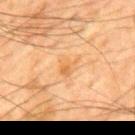No biopsy was performed on this lesion — it was imaged during a full skin examination and was not determined to be concerning.
A roughly 15 mm field-of-view crop from a total-body skin photograph.
The subject is a male aged 63 to 67.
About 3 mm across.
The lesion is located on the mid back.
The total-body-photography lesion software estimated an average lesion color of about L≈54 a*≈19 b*≈38 (CIELAB) and roughly 6 lightness units darker than nearby skin. The analysis additionally found border irregularity of about 3.5 on a 0–10 scale, a within-lesion color-variation index near 3/10, and peripheral color asymmetry of about 1. The software also gave a classifier nevus-likeness of about 0/100.